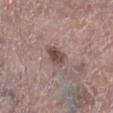No biopsy was performed on this lesion — it was imaged during a full skin examination and was not determined to be concerning.